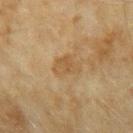Captured during whole-body skin photography for melanoma surveillance; the lesion was not biopsied. Cropped from a whole-body photographic skin survey; the tile spans about 15 mm. On the right upper arm. Imaged with cross-polarized lighting. Approximately 3.5 mm at its widest. A female subject aged 58–62.